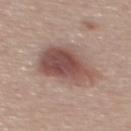Captured during whole-body skin photography for melanoma surveillance; the lesion was not biopsied.
An algorithmic analysis of the crop reported a lesion area of about 24 mm², a shape eccentricity near 0.3, and two-axis asymmetry of about 0.25. It also reported a nevus-likeness score of about 90/100.
The recorded lesion diameter is about 6 mm.
Imaged with white-light lighting.
Cropped from a total-body skin-imaging series; the visible field is about 15 mm.
The lesion is located on the mid back.
A male subject aged approximately 55.